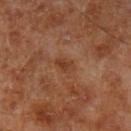The lesion was photographed on a routine skin check and not biopsied; there is no pathology result. The lesion is located on the left lower leg. A male patient, aged 68–72. Longest diameter approximately 2.5 mm. Imaged with cross-polarized lighting. The lesion-visualizer software estimated a lesion-to-skin contrast of about 6.5 (normalized; higher = more distinct). The analysis additionally found a nevus-likeness score of about 0/100. Cropped from a total-body skin-imaging series; the visible field is about 15 mm.